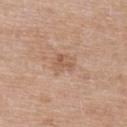Captured during whole-body skin photography for melanoma surveillance; the lesion was not biopsied. Automated tile analysis of the lesion measured a lesion area of about 5 mm², a shape eccentricity near 0.55, and two-axis asymmetry of about 0.25. It also reported an average lesion color of about L≈57 a*≈19 b*≈31 (CIELAB), roughly 8 lightness units darker than nearby skin, and a normalized lesion–skin contrast near 5.5. And it measured a nevus-likeness score of about 0/100. A female subject in their 60s. Approximately 2.5 mm at its widest. A roughly 15 mm field-of-view crop from a total-body skin photograph. Captured under white-light illumination. The lesion is located on the upper back.